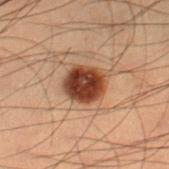Q: Was this lesion biopsied?
A: total-body-photography surveillance lesion; no biopsy
Q: What lighting was used for the tile?
A: cross-polarized
Q: Patient demographics?
A: male, aged around 55
Q: What is the anatomic site?
A: the right lower leg
Q: How large is the lesion?
A: ≈4 mm
Q: What kind of image is this?
A: ~15 mm tile from a whole-body skin photo
Q: Automated lesion metrics?
A: an area of roughly 11 mm²; an average lesion color of about L≈33 a*≈21 b*≈27 (CIELAB), a lesion–skin lightness drop of about 17, and a lesion-to-skin contrast of about 14 (normalized; higher = more distinct); a border-irregularity rating of about 1.5/10 and a within-lesion color-variation index near 5/10; a nevus-likeness score of about 100/100 and a lesion-detection confidence of about 100/100